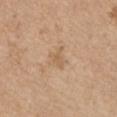The lesion was tiled from a total-body skin photograph and was not biopsied. Captured under white-light illumination. This image is a 15 mm lesion crop taken from a total-body photograph. On the left upper arm. Measured at roughly 3 mm in maximum diameter. A male subject, approximately 65 years of age.